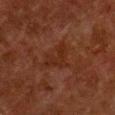{
  "site": "chest",
  "image": {
    "source": "total-body photography crop",
    "field_of_view_mm": 15
  },
  "patient": {
    "sex": "female",
    "age_approx": 50
  }
}